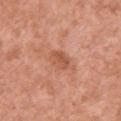The lesion was photographed on a routine skin check and not biopsied; there is no pathology result. The tile uses white-light illumination. The lesion-visualizer software estimated a footprint of about 5.5 mm², a shape eccentricity near 0.75, and a symmetry-axis asymmetry near 0.2. The analysis additionally found a classifier nevus-likeness of about 0/100 and a detector confidence of about 100 out of 100 that the crop contains a lesion. A female patient about 50 years old. A 15 mm close-up extracted from a 3D total-body photography capture. The lesion is on the upper back.